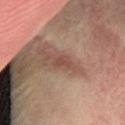Clinical impression: Recorded during total-body skin imaging; not selected for excision or biopsy. Image and clinical context: A male patient aged 63 to 67. Approximately 2.5 mm at its widest. A roughly 15 mm field-of-view crop from a total-body skin photograph. Imaged with cross-polarized lighting. The lesion-visualizer software estimated a footprint of about 3.5 mm² and a shape eccentricity near 0.8. And it measured about 8 CIELAB-L* units darker than the surrounding skin and a lesion-to-skin contrast of about 6 (normalized; higher = more distinct). It also reported a nevus-likeness score of about 0/100 and lesion-presence confidence of about 100/100. From the lower back.